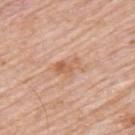follow-up — catalogued during a skin exam; not biopsied
lighting — white-light illumination
TBP lesion metrics — a footprint of about 5 mm² and an eccentricity of roughly 0.8; a border-irregularity index near 3.5/10, a color-variation rating of about 4/10, and peripheral color asymmetry of about 1.5
lesion diameter — about 3.5 mm
image source — ~15 mm tile from a whole-body skin photo
site — the upper back
subject — male, aged around 80